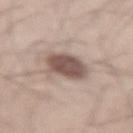Q: Was this lesion biopsied?
A: imaged on a skin check; not biopsied
Q: How was the tile lit?
A: white-light
Q: What are the patient's age and sex?
A: male, about 35 years old
Q: What is the imaging modality?
A: ~15 mm crop, total-body skin-cancer survey
Q: What is the anatomic site?
A: the right thigh
Q: What is the lesion's diameter?
A: about 4 mm